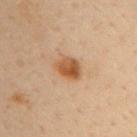Recorded during total-body skin imaging; not selected for excision or biopsy. About 3.5 mm across. The total-body-photography lesion software estimated a lesion area of about 6.5 mm² and a shape-asymmetry score of about 0.2 (0 = symmetric). The analysis additionally found a border-irregularity rating of about 2/10, a within-lesion color-variation index near 6.5/10, and a peripheral color-asymmetry measure near 2.5. The analysis additionally found a nevus-likeness score of about 100/100 and lesion-presence confidence of about 100/100. On the arm. The subject is a male approximately 40 years of age. A close-up tile cropped from a whole-body skin photograph, about 15 mm across.